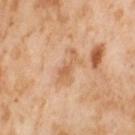Recorded during total-body skin imaging; not selected for excision or biopsy.